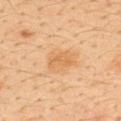| key | value |
|---|---|
| biopsy status | imaged on a skin check; not biopsied |
| acquisition | 15 mm crop, total-body photography |
| tile lighting | cross-polarized |
| patient | male, roughly 35 years of age |
| location | the upper back |
| size | ≈3 mm |